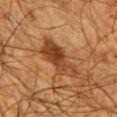Impression:
This lesion was catalogued during total-body skin photography and was not selected for biopsy.